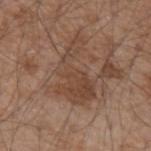Impression:
Captured during whole-body skin photography for melanoma surveillance; the lesion was not biopsied.
Image and clinical context:
The lesion is located on the left forearm. Approximately 7.5 mm at its widest. The tile uses white-light illumination. The subject is a male in their mid- to late 50s. Automated tile analysis of the lesion measured a lesion color around L≈44 a*≈18 b*≈28 in CIELAB, about 8 CIELAB-L* units darker than the surrounding skin, and a normalized lesion–skin contrast near 6. It also reported a border-irregularity rating of about 9/10, a within-lesion color-variation index near 3/10, and peripheral color asymmetry of about 1. And it measured lesion-presence confidence of about 95/100. This image is a 15 mm lesion crop taken from a total-body photograph.